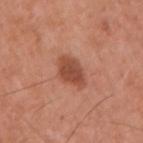Case summary:
• workup — catalogued during a skin exam; not biopsied
• subject — male, aged 53 to 57
• image source — total-body-photography crop, ~15 mm field of view
• size — ≈4 mm
• location — the right upper arm
• image-analysis metrics — a shape eccentricity near 0.8 and a shape-asymmetry score of about 0.2 (0 = symmetric); an average lesion color of about L≈48 a*≈26 b*≈31 (CIELAB); a nevus-likeness score of about 75/100 and a lesion-detection confidence of about 100/100